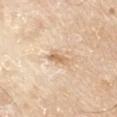Imaged during a routine full-body skin examination; the lesion was not biopsied and no histopathology is available. A male patient roughly 80 years of age. On the left upper arm. A 15 mm close-up tile from a total-body photography series done for melanoma screening.